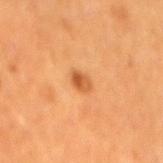lighting: cross-polarized
anatomic site: the lower back
lesion size: about 2.5 mm
image source: ~15 mm tile from a whole-body skin photo
image-analysis metrics: a footprint of about 3 mm² and an eccentricity of roughly 0.65; a lesion color around L≈51 a*≈27 b*≈41 in CIELAB, about 11 CIELAB-L* units darker than the surrounding skin, and a lesion-to-skin contrast of about 7.5 (normalized; higher = more distinct)
subject: male, roughly 65 years of age